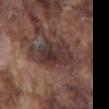Q: Was a biopsy performed?
A: total-body-photography surveillance lesion; no biopsy
Q: What are the patient's age and sex?
A: male, aged approximately 75
Q: What did automated image analysis measure?
A: a lesion color around L≈34 a*≈16 b*≈19 in CIELAB, about 10 CIELAB-L* units darker than the surrounding skin, and a lesion-to-skin contrast of about 9.5 (normalized; higher = more distinct); a border-irregularity rating of about 4.5/10 and peripheral color asymmetry of about 1.5
Q: Where on the body is the lesion?
A: the back
Q: What kind of image is this?
A: total-body-photography crop, ~15 mm field of view
Q: How was the tile lit?
A: white-light illumination